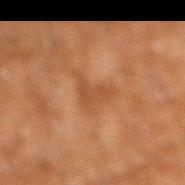This lesion was catalogued during total-body skin photography and was not selected for biopsy.
Measured at roughly 4.5 mm in maximum diameter.
The lesion is located on the right lower leg.
The patient is a male in their 60s.
This image is a 15 mm lesion crop taken from a total-body photograph.
Automated tile analysis of the lesion measured a lesion area of about 6 mm². And it measured about 6 CIELAB-L* units darker than the surrounding skin and a normalized lesion–skin contrast near 5. The software also gave a peripheral color-asymmetry measure near 0.5. It also reported an automated nevus-likeness rating near 0 out of 100.
Captured under cross-polarized illumination.